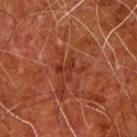follow-up — total-body-photography surveillance lesion; no biopsy.